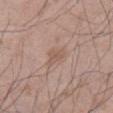Case summary:
– body site: the abdomen
– diameter: ≈2.5 mm
– patient: male, aged 48–52
– image source: ~15 mm tile from a whole-body skin photo
– lighting: white-light
– TBP lesion metrics: a lesion area of about 3.5 mm² and a shape eccentricity near 0.7; a lesion color around L≈56 a*≈17 b*≈27 in CIELAB, about 7 CIELAB-L* units darker than the surrounding skin, and a normalized lesion–skin contrast near 5; a nevus-likeness score of about 0/100 and lesion-presence confidence of about 100/100